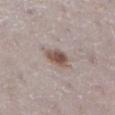This lesion was catalogued during total-body skin photography and was not selected for biopsy. The lesion's longest dimension is about 2.5 mm. A close-up tile cropped from a whole-body skin photograph, about 15 mm across. A female patient, roughly 30 years of age. The lesion is on the right lower leg. The lesion-visualizer software estimated a lesion area of about 5.5 mm² and an eccentricity of roughly 0.55. The analysis additionally found a lesion–skin lightness drop of about 12 and a normalized border contrast of about 9. The software also gave an automated nevus-likeness rating near 95 out of 100. Captured under white-light illumination.